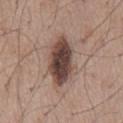{
  "biopsy_status": "not biopsied; imaged during a skin examination",
  "lesion_size": {
    "long_diameter_mm_approx": 6.5
  },
  "site": "mid back",
  "image": {
    "source": "total-body photography crop",
    "field_of_view_mm": 15
  },
  "patient": {
    "sex": "male",
    "age_approx": 55
  },
  "lighting": "white-light"
}